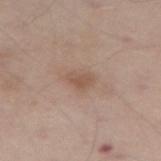Q: Was this lesion biopsied?
A: catalogued during a skin exam; not biopsied
Q: What kind of image is this?
A: 15 mm crop, total-body photography
Q: What are the patient's age and sex?
A: male, about 75 years old
Q: Where on the body is the lesion?
A: the abdomen